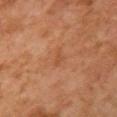  biopsy_status: not biopsied; imaged during a skin examination
  automated_metrics:
    border_irregularity_0_10: 3.5
    color_variation_0_10: 0.0
    peripheral_color_asymmetry: 0.0
  lighting: cross-polarized
  patient:
    sex: female
    age_approx: 60
  site: front of the torso
  image:
    source: total-body photography crop
    field_of_view_mm: 15